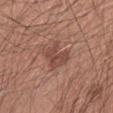The lesion was tiled from a total-body skin photograph and was not biopsied.
From the arm.
Measured at roughly 3 mm in maximum diameter.
Automated tile analysis of the lesion measured a symmetry-axis asymmetry near 0.4. The software also gave a within-lesion color-variation index near 2.5/10 and radial color variation of about 1. The analysis additionally found a nevus-likeness score of about 10/100 and a lesion-detection confidence of about 100/100.
A male subject aged around 30.
A 15 mm close-up extracted from a 3D total-body photography capture.
This is a white-light tile.